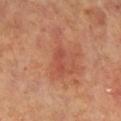Q: Was this lesion biopsied?
A: total-body-photography surveillance lesion; no biopsy
Q: What are the patient's age and sex?
A: female, aged around 70
Q: What is the imaging modality?
A: ~15 mm crop, total-body skin-cancer survey
Q: Automated lesion metrics?
A: an eccentricity of roughly 0.9 and a symmetry-axis asymmetry near 0.4; a lesion color around L≈51 a*≈31 b*≈33 in CIELAB and about 6 CIELAB-L* units darker than the surrounding skin; an automated nevus-likeness rating near 0 out of 100 and lesion-presence confidence of about 100/100
Q: Lesion location?
A: the right lower leg
Q: What lighting was used for the tile?
A: cross-polarized
Q: Lesion size?
A: about 3 mm